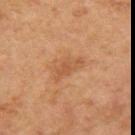Q: Was this lesion biopsied?
A: imaged on a skin check; not biopsied
Q: Where on the body is the lesion?
A: the left upper arm
Q: What lighting was used for the tile?
A: cross-polarized illumination
Q: Who is the patient?
A: female, roughly 60 years of age
Q: How was this image acquired?
A: ~15 mm tile from a whole-body skin photo